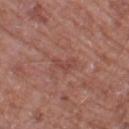Case summary:
– workup · catalogued during a skin exam; not biopsied
– image · ~15 mm crop, total-body skin-cancer survey
– anatomic site · the leg
– patient · male, approximately 60 years of age